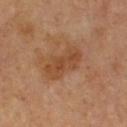| feature | finding |
|---|---|
| biopsy status | imaged on a skin check; not biopsied |
| tile lighting | cross-polarized |
| anatomic site | the chest |
| size | about 5 mm |
| subject | male, in their mid-60s |
| imaging modality | ~15 mm tile from a whole-body skin photo |